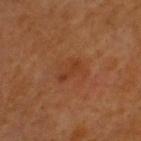Captured during whole-body skin photography for melanoma surveillance; the lesion was not biopsied.
A male patient approximately 60 years of age.
Imaged with cross-polarized lighting.
Located on the head or neck.
Cropped from a whole-body photographic skin survey; the tile spans about 15 mm.